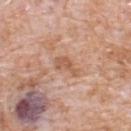workup: no biopsy performed (imaged during a skin exam) | image source: ~15 mm tile from a whole-body skin photo | tile lighting: white-light illumination | lesion size: about 3.5 mm | patient: male, aged approximately 80 | TBP lesion metrics: an area of roughly 4 mm², an outline eccentricity of about 0.9 (0 = round, 1 = elongated), and a symmetry-axis asymmetry near 0.4 | anatomic site: the upper back.